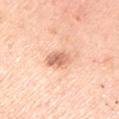• notes — no biopsy performed (imaged during a skin exam)